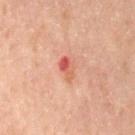Findings:
- workup: imaged on a skin check; not biopsied
- acquisition: total-body-photography crop, ~15 mm field of view
- image-analysis metrics: an average lesion color of about L≈44 a*≈25 b*≈25 (CIELAB) and a normalized border contrast of about 7; a within-lesion color-variation index near 8/10 and a peripheral color-asymmetry measure near 3
- location: the back
- illumination: cross-polarized
- subject: male, aged approximately 70
- lesion size: ≈2.5 mm